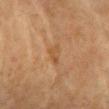This is a cross-polarized tile.
From the chest.
A region of skin cropped from a whole-body photographic capture, roughly 15 mm wide.
A female patient, about 60 years old.
An algorithmic analysis of the crop reported a lesion color around L≈48 a*≈19 b*≈36 in CIELAB, roughly 6 lightness units darker than nearby skin, and a normalized border contrast of about 5. And it measured border irregularity of about 4.5 on a 0–10 scale, a within-lesion color-variation index near 0.5/10, and radial color variation of about 0. The analysis additionally found a classifier nevus-likeness of about 0/100.
Longest diameter approximately 2.5 mm.